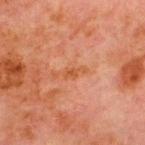Q: Was a biopsy performed?
A: catalogued during a skin exam; not biopsied
Q: Automated lesion metrics?
A: a shape eccentricity near 0.85 and a symmetry-axis asymmetry near 0.45; a lesion color around L≈45 a*≈25 b*≈34 in CIELAB and a lesion–skin lightness drop of about 6; border irregularity of about 5 on a 0–10 scale, a within-lesion color-variation index near 0/10, and radial color variation of about 0; a classifier nevus-likeness of about 0/100
Q: What is the anatomic site?
A: the chest
Q: Patient demographics?
A: male, in their 70s
Q: What kind of image is this?
A: total-body-photography crop, ~15 mm field of view
Q: What lighting was used for the tile?
A: cross-polarized illumination